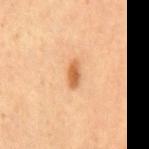No biopsy was performed on this lesion — it was imaged during a full skin examination and was not determined to be concerning. Located on the mid back. The lesion's longest dimension is about 3 mm. The lesion-visualizer software estimated a shape-asymmetry score of about 0.2 (0 = symmetric). And it measured an average lesion color of about L≈60 a*≈25 b*≈41 (CIELAB), a lesion–skin lightness drop of about 13, and a lesion-to-skin contrast of about 8.5 (normalized; higher = more distinct). The analysis additionally found a border-irregularity rating of about 2/10 and a color-variation rating of about 2.5/10. This is a cross-polarized tile. Cropped from a total-body skin-imaging series; the visible field is about 15 mm. The patient is a male aged 68 to 72.